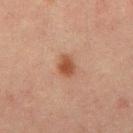Impression:
Imaged during a routine full-body skin examination; the lesion was not biopsied and no histopathology is available.
Background:
The patient is a male aged 48 to 52. An algorithmic analysis of the crop reported a lesion area of about 4.5 mm², a shape eccentricity near 0.75, and a shape-asymmetry score of about 0.35 (0 = symmetric). And it measured an average lesion color of about L≈39 a*≈20 b*≈27 (CIELAB), a lesion–skin lightness drop of about 9, and a lesion-to-skin contrast of about 8.5 (normalized; higher = more distinct). The analysis additionally found border irregularity of about 3 on a 0–10 scale and a within-lesion color-variation index near 2/10. It also reported a classifier nevus-likeness of about 100/100 and lesion-presence confidence of about 100/100. Captured under cross-polarized illumination. Measured at roughly 3 mm in maximum diameter. A 15 mm close-up extracted from a 3D total-body photography capture. The lesion is on the mid back.